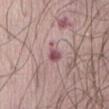From the abdomen. The patient is a male approximately 80 years of age. Cropped from a total-body skin-imaging series; the visible field is about 15 mm. About 2.5 mm across. The tile uses white-light illumination.